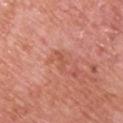Impression: The lesion was tiled from a total-body skin photograph and was not biopsied. Clinical summary: The lesion is on the chest. The subject is a male roughly 70 years of age. Cropped from a total-body skin-imaging series; the visible field is about 15 mm.